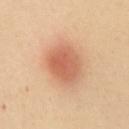Background:
A female patient aged 48 to 52. The lesion is on the front of the torso. A region of skin cropped from a whole-body photographic capture, roughly 15 mm wide.